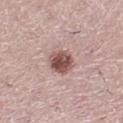Imaged during a routine full-body skin examination; the lesion was not biopsied and no histopathology is available. A female subject, roughly 40 years of age. Longest diameter approximately 3 mm. This is a white-light tile. A close-up tile cropped from a whole-body skin photograph, about 15 mm across. The lesion is located on the right lower leg.